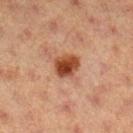No biopsy was performed on this lesion — it was imaged during a full skin examination and was not determined to be concerning.